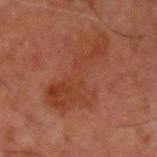Recorded during total-body skin imaging; not selected for excision or biopsy. The recorded lesion diameter is about 8.5 mm. The patient is a male aged around 60. On the left upper arm. A 15 mm close-up tile from a total-body photography series done for melanoma screening.